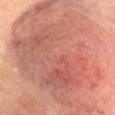Part of a total-body skin-imaging series; this lesion was reviewed on a skin check and was not flagged for biopsy. Longest diameter approximately 21 mm. The total-body-photography lesion software estimated a lesion area of about 130 mm², an outline eccentricity of about 0.85 (0 = round, 1 = elongated), and two-axis asymmetry of about 0.3. It also reported a mean CIELAB color near L≈60 a*≈26 b*≈29, a lesion–skin lightness drop of about 11, and a normalized border contrast of about 7.5. Located on the head or neck. The patient is a female about 75 years old. The tile uses cross-polarized illumination. A roughly 15 mm field-of-view crop from a total-body skin photograph.